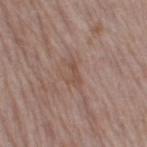<tbp_lesion>
<biopsy_status>not biopsied; imaged during a skin examination</biopsy_status>
<lesion_size>
  <long_diameter_mm_approx>2.5</long_diameter_mm_approx>
</lesion_size>
<lighting>white-light</lighting>
<site>right thigh</site>
<image>
  <source>total-body photography crop</source>
  <field_of_view_mm>15</field_of_view_mm>
</image>
<patient>
  <sex>female</sex>
  <age_approx>40</age_approx>
</patient>
</tbp_lesion>